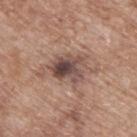Impression:
Imaged during a routine full-body skin examination; the lesion was not biopsied and no histopathology is available.
Background:
A region of skin cropped from a whole-body photographic capture, roughly 15 mm wide. The patient is a male aged 63–67. From the upper back.